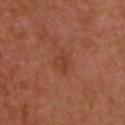Clinical impression:
Recorded during total-body skin imaging; not selected for excision or biopsy.
Context:
The tile uses cross-polarized illumination. The patient is a female aged around 40. On the upper back. Measured at roughly 2.5 mm in maximum diameter. A close-up tile cropped from a whole-body skin photograph, about 15 mm across. An algorithmic analysis of the crop reported a lesion color around L≈42 a*≈27 b*≈33 in CIELAB and a normalized border contrast of about 5. The analysis additionally found a color-variation rating of about 2.5/10 and a peripheral color-asymmetry measure near 1.